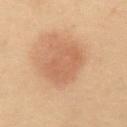{"biopsy_status": "not biopsied; imaged during a skin examination", "site": "abdomen", "automated_metrics": {"cielab_L": 49, "cielab_a": 18, "cielab_b": 29, "vs_skin_darker_L": 7.0, "vs_skin_contrast_norm": 5.0, "border_irregularity_0_10": 2.0, "color_variation_0_10": 2.0, "peripheral_color_asymmetry": 0.5}, "image": {"source": "total-body photography crop", "field_of_view_mm": 15}, "lesion_size": {"long_diameter_mm_approx": 5.0}, "patient": {"sex": "male", "age_approx": 55}}